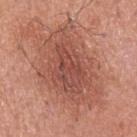Measured at roughly 9.5 mm in maximum diameter.
Captured under white-light illumination.
A 15 mm close-up tile from a total-body photography series done for melanoma screening.
From the arm.
A male subject in their mid- to late 50s.